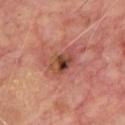The lesion was photographed on a routine skin check and not biopsied; there is no pathology result. The recorded lesion diameter is about 3.5 mm. A lesion tile, about 15 mm wide, cut from a 3D total-body photograph. This is a cross-polarized tile. A male patient, aged approximately 65. Located on the chest.